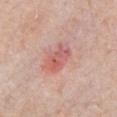{
  "biopsy_status": "not biopsied; imaged during a skin examination",
  "image": {
    "source": "total-body photography crop",
    "field_of_view_mm": 15
  },
  "lighting": "white-light",
  "patient": {
    "sex": "male",
    "age_approx": 75
  },
  "site": "chest"
}